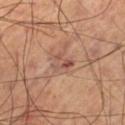This lesion was catalogued during total-body skin photography and was not selected for biopsy.
A 15 mm close-up extracted from a 3D total-body photography capture.
The total-body-photography lesion software estimated a lesion color around L≈50 a*≈21 b*≈26 in CIELAB, about 8 CIELAB-L* units darker than the surrounding skin, and a lesion-to-skin contrast of about 6.5 (normalized; higher = more distinct). It also reported a border-irregularity rating of about 4.5/10, a color-variation rating of about 7.5/10, and peripheral color asymmetry of about 2.5.
Located on the left thigh.
The patient is a male aged 68 to 72.
Measured at roughly 3 mm in maximum diameter.